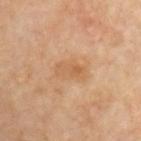Imaged during a routine full-body skin examination; the lesion was not biopsied and no histopathology is available.
This is a cross-polarized tile.
From the front of the torso.
A region of skin cropped from a whole-body photographic capture, roughly 15 mm wide.
An algorithmic analysis of the crop reported roughly 6 lightness units darker than nearby skin and a lesion-to-skin contrast of about 5 (normalized; higher = more distinct). And it measured a peripheral color-asymmetry measure near 1.
Approximately 3.5 mm at its widest.
The patient is a male in their mid-50s.